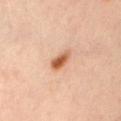<case>
<biopsy_status>not biopsied; imaged during a skin examination</biopsy_status>
<site>arm</site>
<lesion_size>
  <long_diameter_mm_approx>3.0</long_diameter_mm_approx>
</lesion_size>
<lighting>cross-polarized</lighting>
<patient>
  <sex>male</sex>
  <age_approx>45</age_approx>
</patient>
<image>
  <source>total-body photography crop</source>
  <field_of_view_mm>15</field_of_view_mm>
</image>
</case>